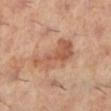Clinical impression: The lesion was tiled from a total-body skin photograph and was not biopsied. Image and clinical context: Captured under cross-polarized illumination. Cropped from a whole-body photographic skin survey; the tile spans about 15 mm. A female patient roughly 60 years of age. On the left lower leg. Measured at roughly 6 mm in maximum diameter.